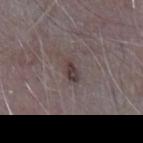notes: imaged on a skin check; not biopsied | diameter: about 3 mm | automated lesion analysis: an eccentricity of roughly 0.8 and a symmetry-axis asymmetry near 0.3 | patient: male, approximately 65 years of age | location: the abdomen | imaging modality: ~15 mm tile from a whole-body skin photo.